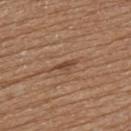No biopsy was performed on this lesion — it was imaged during a full skin examination and was not determined to be concerning.
On the upper back.
A female subject aged 73 to 77.
The lesion-visualizer software estimated an eccentricity of roughly 0.9 and a shape-asymmetry score of about 0.3 (0 = symmetric). The software also gave a nevus-likeness score of about 0/100 and a lesion-detection confidence of about 65/100.
A 15 mm crop from a total-body photograph taken for skin-cancer surveillance.